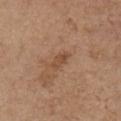Notes:
* biopsy status: imaged on a skin check; not biopsied
* lesion size: ~3 mm (longest diameter)
* automated metrics: an area of roughly 3 mm², an eccentricity of roughly 0.9, and two-axis asymmetry of about 0.35; a lesion color around L≈48 a*≈20 b*≈32 in CIELAB, a lesion–skin lightness drop of about 8, and a normalized border contrast of about 6; a nevus-likeness score of about 0/100 and a lesion-detection confidence of about 100/100
* image: 15 mm crop, total-body photography
* subject: female, aged 63 to 67
* location: the chest